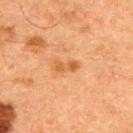Q: Is there a histopathology result?
A: total-body-photography surveillance lesion; no biopsy
Q: Patient demographics?
A: male, aged 48–52
Q: What is the anatomic site?
A: the upper back
Q: What is the imaging modality?
A: ~15 mm tile from a whole-body skin photo